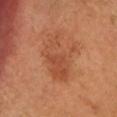Background: A female patient, aged 63 to 67. A lesion tile, about 15 mm wide, cut from a 3D total-body photograph. The lesion is on the head or neck.